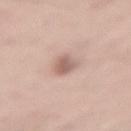<record>
  <biopsy_status>not biopsied; imaged during a skin examination</biopsy_status>
  <lesion_size>
    <long_diameter_mm_approx>3.5</long_diameter_mm_approx>
  </lesion_size>
  <patient>
    <sex>male</sex>
    <age_approx>55</age_approx>
  </patient>
  <site>lower back</site>
  <image>
    <source>total-body photography crop</source>
    <field_of_view_mm>15</field_of_view_mm>
  </image>
  <lighting>white-light</lighting>
</record>